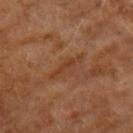workup: total-body-photography surveillance lesion; no biopsy.